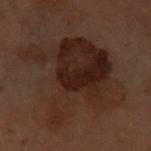workup = catalogued during a skin exam; not biopsied
acquisition = 15 mm crop, total-body photography
patient = female, roughly 60 years of age
lesion size = ≈10 mm
illumination = cross-polarized illumination
anatomic site = the front of the torso
automated lesion analysis = a lesion color around L≈20 a*≈15 b*≈20 in CIELAB, about 7 CIELAB-L* units darker than the surrounding skin, and a normalized lesion–skin contrast near 8.5; a border-irregularity index near 7/10, internal color variation of about 5.5 on a 0–10 scale, and radial color variation of about 1.5